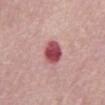This lesion was catalogued during total-body skin photography and was not selected for biopsy.
A roughly 15 mm field-of-view crop from a total-body skin photograph.
The lesion-visualizer software estimated a lesion area of about 7 mm², an outline eccentricity of about 0.65 (0 = round, 1 = elongated), and a shape-asymmetry score of about 0.2 (0 = symmetric). The analysis additionally found an average lesion color of about L≈49 a*≈34 b*≈19 (CIELAB), roughly 18 lightness units darker than nearby skin, and a normalized border contrast of about 12.
Longest diameter approximately 3.5 mm.
The patient is a male approximately 65 years of age.
On the abdomen.